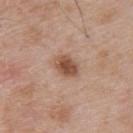The lesion was tiled from a total-body skin photograph and was not biopsied.
Located on the upper back.
Automated image analysis of the tile measured a footprint of about 6 mm², a shape eccentricity near 0.65, and a symmetry-axis asymmetry near 0.2. It also reported a lesion color around L≈51 a*≈21 b*≈30 in CIELAB, roughly 13 lightness units darker than nearby skin, and a normalized lesion–skin contrast near 9.5. The software also gave a border-irregularity index near 2/10 and peripheral color asymmetry of about 1.
A roughly 15 mm field-of-view crop from a total-body skin photograph.
The recorded lesion diameter is about 3 mm.
The tile uses white-light illumination.
The subject is a male aged approximately 55.